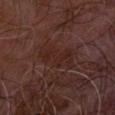Imaged during a routine full-body skin examination; the lesion was not biopsied and no histopathology is available. The lesion is located on the left forearm. Approximately 5 mm at its widest. A 15 mm close-up extracted from a 3D total-body photography capture. Imaged with cross-polarized lighting. The subject is a male about 65 years old.